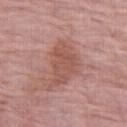Measured at roughly 5 mm in maximum diameter.
This is a white-light tile.
A female subject, in their mid-60s.
From the right thigh.
Cropped from a whole-body photographic skin survey; the tile spans about 15 mm.
Automated image analysis of the tile measured a lesion area of about 12 mm², an eccentricity of roughly 0.8, and two-axis asymmetry of about 0.3.